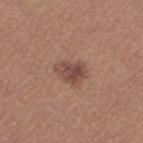Case summary:
– notes · catalogued during a skin exam; not biopsied
– anatomic site · the right thigh
– automated metrics · roughly 10 lightness units darker than nearby skin and a normalized border contrast of about 8; a classifier nevus-likeness of about 70/100 and a lesion-detection confidence of about 100/100
– subject · female, aged approximately 45
– image · ~15 mm tile from a whole-body skin photo
– tile lighting · white-light illumination
– size · ≈3.5 mm